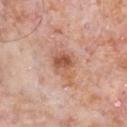A lesion tile, about 15 mm wide, cut from a 3D total-body photograph.
A male patient, aged around 80.
Automated image analysis of the tile measured an outline eccentricity of about 0.8 (0 = round, 1 = elongated) and a symmetry-axis asymmetry near 0.2.
Captured under white-light illumination.
The lesion is located on the front of the torso.